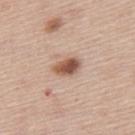Impression:
Captured during whole-body skin photography for melanoma surveillance; the lesion was not biopsied.
Image and clinical context:
A male subject aged approximately 45. Located on the mid back. Cropped from a total-body skin-imaging series; the visible field is about 15 mm. The tile uses white-light illumination.